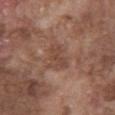The lesion's longest dimension is about 3 mm. A male subject, roughly 75 years of age. This is a white-light tile. This image is a 15 mm lesion crop taken from a total-body photograph. From the front of the torso. Automated image analysis of the tile measured an area of roughly 6 mm², a shape eccentricity near 0.75, and a symmetry-axis asymmetry near 0.3. The analysis additionally found a lesion color around L≈45 a*≈19 b*≈26 in CIELAB, about 7 CIELAB-L* units darker than the surrounding skin, and a normalized lesion–skin contrast near 5.5. It also reported a border-irregularity index near 3.5/10 and peripheral color asymmetry of about 0.5.